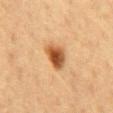The lesion was tiled from a total-body skin photograph and was not biopsied. A male subject, in their 60s. The lesion is located on the chest. The total-body-photography lesion software estimated roughly 15 lightness units darker than nearby skin. It also reported a border-irregularity rating of about 2.5/10 and radial color variation of about 1.5. The software also gave an automated nevus-likeness rating near 100 out of 100 and a detector confidence of about 100 out of 100 that the crop contains a lesion. This image is a 15 mm lesion crop taken from a total-body photograph. Imaged with cross-polarized lighting. Approximately 3 mm at its widest.